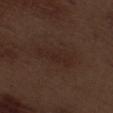Clinical impression:
Captured during whole-body skin photography for melanoma surveillance; the lesion was not biopsied.
Clinical summary:
From the leg. A male patient aged approximately 70. A region of skin cropped from a whole-body photographic capture, roughly 15 mm wide. This is a white-light tile. Longest diameter approximately 5 mm.